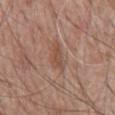{
  "biopsy_status": "not biopsied; imaged during a skin examination",
  "image": {
    "source": "total-body photography crop",
    "field_of_view_mm": 15
  },
  "lighting": "white-light",
  "site": "mid back",
  "patient": {
    "sex": "male",
    "age_approx": 60
  },
  "lesion_size": {
    "long_diameter_mm_approx": 4.0
  }
}